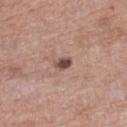Case summary:
* workup: imaged on a skin check; not biopsied
* acquisition: 15 mm crop, total-body photography
* location: the right lower leg
* lighting: white-light illumination
* patient: female, in their 70s
* automated lesion analysis: an eccentricity of roughly 0.7 and a shape-asymmetry score of about 0.15 (0 = symmetric); an average lesion color of about L≈48 a*≈18 b*≈22 (CIELAB), roughly 14 lightness units darker than nearby skin, and a normalized lesion–skin contrast near 10; a border-irregularity rating of about 1.5/10, a color-variation rating of about 4/10, and a peripheral color-asymmetry measure near 1.5; a classifier nevus-likeness of about 75/100 and lesion-presence confidence of about 100/100
* lesion size: ~2.5 mm (longest diameter)